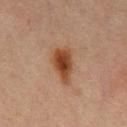Clinical impression: Recorded during total-body skin imaging; not selected for excision or biopsy. Image and clinical context: From the mid back. Measured at roughly 5 mm in maximum diameter. The subject is a male about 70 years old. A roughly 15 mm field-of-view crop from a total-body skin photograph.